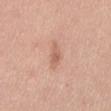| field | value |
|---|---|
| patient | male, about 30 years old |
| size | ~4 mm (longest diameter) |
| lighting | white-light illumination |
| acquisition | ~15 mm crop, total-body skin-cancer survey |
| site | the mid back |